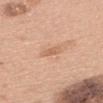<record>
  <biopsy_status>not biopsied; imaged during a skin examination</biopsy_status>
  <patient>
    <sex>female</sex>
    <age_approx>60</age_approx>
  </patient>
  <lesion_size>
    <long_diameter_mm_approx>3.0</long_diameter_mm_approx>
  </lesion_size>
  <site>upper back</site>
  <lighting>white-light</lighting>
  <automated_metrics>
    <shape_asymmetry>0.3</shape_asymmetry>
    <cielab_L>63</cielab_L>
    <cielab_a>22</cielab_a>
    <cielab_b>34</cielab_b>
    <vs_skin_darker_L>8.0</vs_skin_darker_L>
    <vs_skin_contrast_norm>5.5</vs_skin_contrast_norm>
  </automated_metrics>
  <image>
    <source>total-body photography crop</source>
    <field_of_view_mm>15</field_of_view_mm>
  </image>
</record>